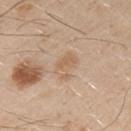Context:
A male subject, about 30 years old. A 15 mm close-up tile from a total-body photography series done for melanoma screening. Imaged with white-light lighting. The recorded lesion diameter is about 3 mm. Automated tile analysis of the lesion measured roughly 7 lightness units darker than nearby skin and a normalized lesion–skin contrast near 5.5. It also reported border irregularity of about 3.5 on a 0–10 scale, a color-variation rating of about 2/10, and peripheral color asymmetry of about 0.5. The lesion is located on the left upper arm.